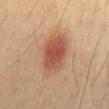The lesion was photographed on a routine skin check and not biopsied; there is no pathology result.
The patient is a male approximately 40 years of age.
The lesion-visualizer software estimated a lesion color around L≈46 a*≈21 b*≈29 in CIELAB and a normalized lesion–skin contrast near 8.5. The software also gave border irregularity of about 2 on a 0–10 scale, a color-variation rating of about 5/10, and radial color variation of about 1.5.
Longest diameter approximately 5.5 mm.
This is a cross-polarized tile.
A 15 mm close-up tile from a total-body photography series done for melanoma screening.
From the front of the torso.